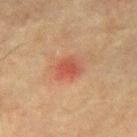<tbp_lesion>
  <biopsy_status>not biopsied; imaged during a skin examination</biopsy_status>
  <lesion_size>
    <long_diameter_mm_approx>3.0</long_diameter_mm_approx>
  </lesion_size>
  <lighting>cross-polarized</lighting>
  <image>
    <source>total-body photography crop</source>
    <field_of_view_mm>15</field_of_view_mm>
  </image>
  <patient>
    <sex>male</sex>
    <age_approx>75</age_approx>
  </patient>
  <site>mid back</site>
</tbp_lesion>